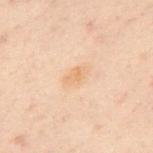  patient:
    sex: male
    age_approx: 50
  site: mid back
  lesion_size:
    long_diameter_mm_approx: 3.5
  image:
    source: total-body photography crop
    field_of_view_mm: 15
  automated_metrics:
    area_mm2_approx: 5.0
    eccentricity: 0.8
    shape_asymmetry: 0.15
    nevus_likeness_0_100: 0
    lesion_detection_confidence_0_100: 100